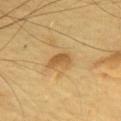Imaged during a routine full-body skin examination; the lesion was not biopsied and no histopathology is available. A male patient aged around 65. Approximately 3 mm at its widest. Automated tile analysis of the lesion measured an area of roughly 4.5 mm², a shape eccentricity near 0.8, and a symmetry-axis asymmetry near 0.4. The analysis additionally found an average lesion color of about L≈58 a*≈18 b*≈43 (CIELAB), a lesion–skin lightness drop of about 10, and a lesion-to-skin contrast of about 7 (normalized; higher = more distinct). The software also gave an automated nevus-likeness rating near 55 out of 100 and a lesion-detection confidence of about 100/100. Captured under cross-polarized illumination. From the chest. Cropped from a total-body skin-imaging series; the visible field is about 15 mm.